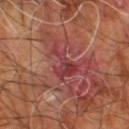patient=male, aged approximately 60 | anatomic site=the right leg | acquisition=~15 mm tile from a whole-body skin photo | lesion diameter=≈5 mm | pathology=a superficial basal cell carcinoma (malignant).